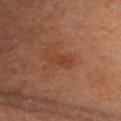Background: Cropped from a whole-body photographic skin survey; the tile spans about 15 mm. About 2.5 mm across. Imaged with cross-polarized lighting. The subject is a male aged 58 to 62. The lesion is on the chest.